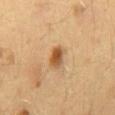Impression: Recorded during total-body skin imaging; not selected for excision or biopsy. Clinical summary: From the back. The total-body-photography lesion software estimated an average lesion color of about L≈44 a*≈17 b*≈33 (CIELAB) and roughly 11 lightness units darker than nearby skin. The software also gave a border-irregularity rating of about 2/10 and a color-variation rating of about 4/10. And it measured an automated nevus-likeness rating near 100 out of 100. A female patient, aged around 40. The recorded lesion diameter is about 3 mm. Imaged with cross-polarized lighting. Cropped from a whole-body photographic skin survey; the tile spans about 15 mm.